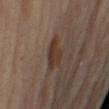- notes · imaged on a skin check; not biopsied